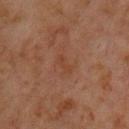A male patient in their 60s. This is a cross-polarized tile. From the chest. Measured at roughly 2.5 mm in maximum diameter. A roughly 15 mm field-of-view crop from a total-body skin photograph. The lesion-visualizer software estimated an area of roughly 2.5 mm². The analysis additionally found about 4 CIELAB-L* units darker than the surrounding skin and a lesion-to-skin contrast of about 5 (normalized; higher = more distinct).